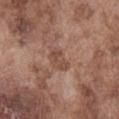biopsy_status: not biopsied; imaged during a skin examination
patient:
  sex: male
  age_approx: 75
lesion_size:
  long_diameter_mm_approx: 3.0
site: abdomen
image:
  source: total-body photography crop
  field_of_view_mm: 15
lighting: white-light